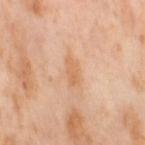Captured during whole-body skin photography for melanoma surveillance; the lesion was not biopsied. A 15 mm close-up extracted from a 3D total-body photography capture. The tile uses cross-polarized illumination. A female subject aged around 55. Located on the right thigh. Measured at roughly 3 mm in maximum diameter.